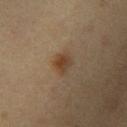Impression: This lesion was catalogued during total-body skin photography and was not selected for biopsy. Clinical summary: A close-up tile cropped from a whole-body skin photograph, about 15 mm across. A male subject, aged 38–42. From the right upper arm. About 2.5 mm across. The lesion-visualizer software estimated an average lesion color of about L≈36 a*≈14 b*≈27 (CIELAB), a lesion–skin lightness drop of about 7, and a lesion-to-skin contrast of about 7.5 (normalized; higher = more distinct). Imaged with cross-polarized lighting.